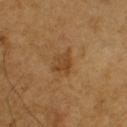{"biopsy_status": "not biopsied; imaged during a skin examination", "lighting": "cross-polarized", "image": {"source": "total-body photography crop", "field_of_view_mm": 15}, "automated_metrics": {"area_mm2_approx": 4.5, "eccentricity": 0.6, "shape_asymmetry": 0.3}, "site": "left upper arm", "patient": {"sex": "male", "age_approx": 65}}